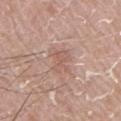| feature | finding |
|---|---|
| workup | total-body-photography surveillance lesion; no biopsy |
| location | the right upper arm |
| image-analysis metrics | an average lesion color of about L≈58 a*≈19 b*≈26 (CIELAB), roughly 6 lightness units darker than nearby skin, and a lesion-to-skin contrast of about 4.5 (normalized; higher = more distinct); a border-irregularity index near 4/10, internal color variation of about 2 on a 0–10 scale, and radial color variation of about 0.5; an automated nevus-likeness rating near 0 out of 100 and a detector confidence of about 100 out of 100 that the crop contains a lesion |
| subject | male, approximately 65 years of age |
| imaging modality | 15 mm crop, total-body photography |